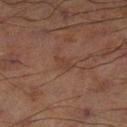Case summary:
• notes — catalogued during a skin exam; not biopsied
• automated lesion analysis — border irregularity of about 3 on a 0–10 scale and a color-variation rating of about 0.5/10; an automated nevus-likeness rating near 0 out of 100 and lesion-presence confidence of about 100/100
• subject — male, aged 68 to 72
• lesion size — ~2.5 mm (longest diameter)
• acquisition — 15 mm crop, total-body photography
• site — the left lower leg
• lighting — cross-polarized